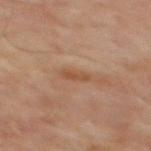The lesion was tiled from a total-body skin photograph and was not biopsied.
The subject is a male in their mid-60s.
Automated tile analysis of the lesion measured roughly 7 lightness units darker than nearby skin. The software also gave an automated nevus-likeness rating near 0 out of 100 and a lesion-detection confidence of about 100/100.
Measured at roughly 2.5 mm in maximum diameter.
A 15 mm close-up tile from a total-body photography series done for melanoma screening.
On the mid back.
Captured under cross-polarized illumination.